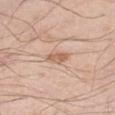Q: Was this lesion biopsied?
A: no biopsy performed (imaged during a skin exam)
Q: What is the imaging modality?
A: total-body-photography crop, ~15 mm field of view
Q: What is the lesion's diameter?
A: ~2.5 mm (longest diameter)
Q: What is the anatomic site?
A: the right thigh
Q: What are the patient's age and sex?
A: male, roughly 55 years of age